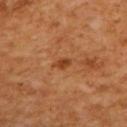Imaged during a routine full-body skin examination; the lesion was not biopsied and no histopathology is available.
The lesion is located on the upper back.
An algorithmic analysis of the crop reported a border-irregularity rating of about 3/10 and a within-lesion color-variation index near 2/10. The software also gave a nevus-likeness score of about 0/100 and a lesion-detection confidence of about 100/100.
This is a cross-polarized tile.
This image is a 15 mm lesion crop taken from a total-body photograph.
A male subject in their mid-60s.